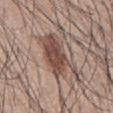biopsy_status: not biopsied; imaged during a skin examination
image:
  source: total-body photography crop
  field_of_view_mm: 15
patient:
  sex: male
  age_approx: 45
automated_metrics:
  cielab_L: 48
  cielab_a: 18
  cielab_b: 24
  vs_skin_darker_L: 13.0
  nevus_likeness_0_100: 100
  lesion_detection_confidence_0_100: 100
site: mid back
lesion_size:
  long_diameter_mm_approx: 7.0
lighting: white-light